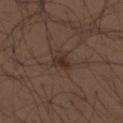- workup: total-body-photography surveillance lesion; no biopsy
- site: the upper back
- subject: male, approximately 30 years of age
- imaging modality: 15 mm crop, total-body photography